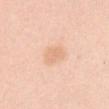The lesion was photographed on a routine skin check and not biopsied; there is no pathology result. About 2.5 mm across. The lesion is on the front of the torso. This is a white-light tile. A female subject aged 38 to 42. Cropped from a whole-body photographic skin survey; the tile spans about 15 mm. An algorithmic analysis of the crop reported a shape eccentricity near 0.75. It also reported a lesion color around L≈74 a*≈21 b*≈35 in CIELAB, a lesion–skin lightness drop of about 7, and a lesion-to-skin contrast of about 5 (normalized; higher = more distinct). The analysis additionally found a nevus-likeness score of about 5/100 and a lesion-detection confidence of about 100/100.